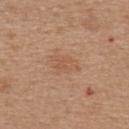Impression:
Part of a total-body skin-imaging series; this lesion was reviewed on a skin check and was not flagged for biopsy.
Background:
Longest diameter approximately 3 mm. This is a white-light tile. On the upper back. A female subject aged 43–47. The lesion-visualizer software estimated a lesion color around L≈55 a*≈21 b*≈32 in CIELAB and a lesion–skin lightness drop of about 6. A close-up tile cropped from a whole-body skin photograph, about 15 mm across.